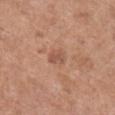notes = no biopsy performed (imaged during a skin exam).